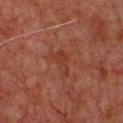Notes:
- workup: no biopsy performed (imaged during a skin exam)
- image source: ~15 mm crop, total-body skin-cancer survey
- subject: male, aged 58–62
- illumination: cross-polarized illumination
- automated lesion analysis: a footprint of about 5.5 mm², an outline eccentricity of about 0.75 (0 = round, 1 = elongated), and a symmetry-axis asymmetry near 0.6; an average lesion color of about L≈28 a*≈21 b*≈24 (CIELAB) and a normalized lesion–skin contrast near 5.5; border irregularity of about 6 on a 0–10 scale, a within-lesion color-variation index near 1.5/10, and peripheral color asymmetry of about 0.5; a nevus-likeness score of about 0/100 and lesion-presence confidence of about 100/100
- site: the chest
- size: ~3.5 mm (longest diameter)